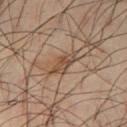Imaged during a routine full-body skin examination; the lesion was not biopsied and no histopathology is available. The lesion is on the left thigh. A male patient, aged around 50. A close-up tile cropped from a whole-body skin photograph, about 15 mm across. The lesion's longest dimension is about 3.5 mm. Imaged with cross-polarized lighting.